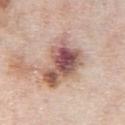Assessment: No biopsy was performed on this lesion — it was imaged during a full skin examination and was not determined to be concerning. Context: The lesion is located on the chest. A male patient, in their mid- to late 70s. The lesion's longest dimension is about 7.5 mm. A roughly 15 mm field-of-view crop from a total-body skin photograph. This is a white-light tile.